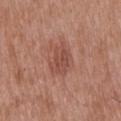Assessment:
The lesion was tiled from a total-body skin photograph and was not biopsied.